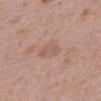workup = total-body-photography surveillance lesion; no biopsy
subject = female, approximately 40 years of age
tile lighting = white-light illumination
site = the abdomen
lesion size = ≈3 mm
image = total-body-photography crop, ~15 mm field of view